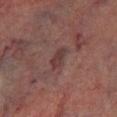patient = male, in their mid- to late 70s | image source = ~15 mm crop, total-body skin-cancer survey | body site = the left lower leg.